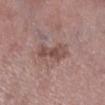The lesion was tiled from a total-body skin photograph and was not biopsied. From the right lower leg. Imaged with white-light lighting. A lesion tile, about 15 mm wide, cut from a 3D total-body photograph. Approximately 4 mm at its widest. A male subject about 70 years old.